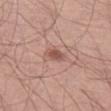<lesion>
<biopsy_status>not biopsied; imaged during a skin examination</biopsy_status>
<site>leg</site>
<image>
  <source>total-body photography crop</source>
  <field_of_view_mm>15</field_of_view_mm>
</image>
<patient>
  <sex>male</sex>
  <age_approx>70</age_approx>
</patient>
</lesion>